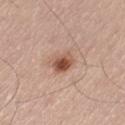biopsy_status: not biopsied; imaged during a skin examination
image:
  source: total-body photography crop
  field_of_view_mm: 15
site: left thigh
lesion_size:
  long_diameter_mm_approx: 2.5
patient:
  sex: male
  age_approx: 60
lighting: white-light